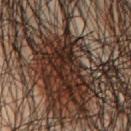Case summary:
– notes — imaged on a skin check; not biopsied
– imaging modality — ~15 mm crop, total-body skin-cancer survey
– TBP lesion metrics — a lesion area of about 15 mm², a shape eccentricity near 0.9, and two-axis asymmetry of about 0.55
– lesion diameter — about 8 mm
– patient — male, roughly 45 years of age
– location — the chest